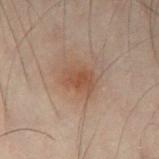Impression: Imaged during a routine full-body skin examination; the lesion was not biopsied and no histopathology is available. Clinical summary: The recorded lesion diameter is about 3.5 mm. The lesion is located on the left thigh. The lesion-visualizer software estimated border irregularity of about 2 on a 0–10 scale, a color-variation rating of about 2.5/10, and a peripheral color-asymmetry measure near 0.5. The software also gave a classifier nevus-likeness of about 45/100 and a lesion-detection confidence of about 100/100. The subject is a male aged approximately 50. A close-up tile cropped from a whole-body skin photograph, about 15 mm across. This is a cross-polarized tile.